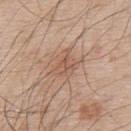{
  "site": "upper back",
  "patient": {
    "sex": "male",
    "age_approx": 55
  },
  "image": {
    "source": "total-body photography crop",
    "field_of_view_mm": 15
  }
}